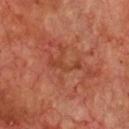workup — imaged on a skin check; not biopsied | image source — total-body-photography crop, ~15 mm field of view | subject — male, in their 70s | automated metrics — an area of roughly 4 mm² and a shape eccentricity near 0.95; a mean CIELAB color near L≈42 a*≈28 b*≈34, about 6 CIELAB-L* units darker than the surrounding skin, and a normalized border contrast of about 5.5; a nevus-likeness score of about 0/100 | size — ≈4 mm | anatomic site — the chest.